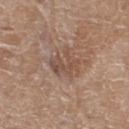No biopsy was performed on this lesion — it was imaged during a full skin examination and was not determined to be concerning. The tile uses white-light illumination. The subject is a female about 75 years old. A region of skin cropped from a whole-body photographic capture, roughly 15 mm wide. Measured at roughly 3.5 mm in maximum diameter. On the right thigh.